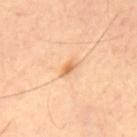{"biopsy_status": "not biopsied; imaged during a skin examination", "site": "mid back", "image": {"source": "total-body photography crop", "field_of_view_mm": 15}, "patient": {"sex": "male", "age_approx": 60}}